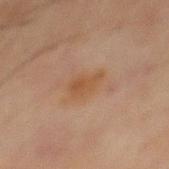Recorded during total-body skin imaging; not selected for excision or biopsy. Automated image analysis of the tile measured a footprint of about 5.5 mm² and two-axis asymmetry of about 0.35. It also reported a mean CIELAB color near L≈42 a*≈17 b*≈29, a lesion–skin lightness drop of about 5, and a normalized border contrast of about 6.5. The software also gave border irregularity of about 3.5 on a 0–10 scale, a within-lesion color-variation index near 2/10, and radial color variation of about 1. Captured under cross-polarized illumination. From the abdomen. A roughly 15 mm field-of-view crop from a total-body skin photograph. A male patient approximately 70 years of age. Longest diameter approximately 3.5 mm.